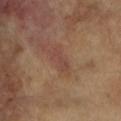Findings:
* biopsy status: no biopsy performed (imaged during a skin exam)
* acquisition: total-body-photography crop, ~15 mm field of view
* automated lesion analysis: a shape eccentricity near 0.9 and a symmetry-axis asymmetry near 0.35; a lesion color around L≈43 a*≈21 b*≈26 in CIELAB and about 6 CIELAB-L* units darker than the surrounding skin; a border-irregularity index near 4/10 and peripheral color asymmetry of about 0; a classifier nevus-likeness of about 0/100 and a lesion-detection confidence of about 95/100
* location: the right forearm
* patient: female, about 75 years old